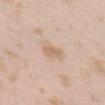Impression:
Part of a total-body skin-imaging series; this lesion was reviewed on a skin check and was not flagged for biopsy.
Background:
A 15 mm crop from a total-body photograph taken for skin-cancer surveillance. A female subject, aged approximately 25. From the head or neck.